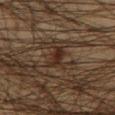No biopsy was performed on this lesion — it was imaged during a full skin examination and was not determined to be concerning. A male patient, aged around 50. A 15 mm close-up extracted from a 3D total-body photography capture. The lesion's longest dimension is about 3.5 mm. Automated tile analysis of the lesion measured an area of roughly 4.5 mm², an outline eccentricity of about 0.9 (0 = round, 1 = elongated), and two-axis asymmetry of about 0.35. The software also gave a lesion color around L≈24 a*≈15 b*≈22 in CIELAB and a lesion-to-skin contrast of about 8 (normalized; higher = more distinct). The analysis additionally found border irregularity of about 3.5 on a 0–10 scale. The software also gave an automated nevus-likeness rating near 10 out of 100. On the chest. This is a cross-polarized tile.